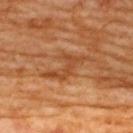Q: Patient demographics?
A: female, in their mid-60s
Q: How was the tile lit?
A: cross-polarized
Q: What did automated image analysis measure?
A: a mean CIELAB color near L≈47 a*≈26 b*≈39 and a lesion-to-skin contrast of about 6.5 (normalized; higher = more distinct); border irregularity of about 9 on a 0–10 scale, internal color variation of about 2 on a 0–10 scale, and peripheral color asymmetry of about 0.5
Q: What is the anatomic site?
A: the back
Q: How was this image acquired?
A: ~15 mm tile from a whole-body skin photo